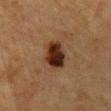notes: imaged on a skin check; not biopsied
tile lighting: cross-polarized
imaging modality: total-body-photography crop, ~15 mm field of view
patient: female, in their mid-50s
automated lesion analysis: roughly 14 lightness units darker than nearby skin and a lesion-to-skin contrast of about 14 (normalized; higher = more distinct); a border-irregularity rating of about 1.5/10, a color-variation rating of about 5.5/10, and radial color variation of about 2; a nevus-likeness score of about 100/100 and a lesion-detection confidence of about 100/100
anatomic site: the chest